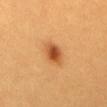Captured during whole-body skin photography for melanoma surveillance; the lesion was not biopsied.
A female patient aged 28–32.
An algorithmic analysis of the crop reported a footprint of about 7 mm² and an outline eccentricity of about 0.7 (0 = round, 1 = elongated). It also reported a border-irregularity rating of about 1.5/10.
A 15 mm close-up extracted from a 3D total-body photography capture.
Captured under cross-polarized illumination.
On the abdomen.
Measured at roughly 3.5 mm in maximum diameter.